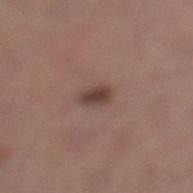  biopsy_status: not biopsied; imaged during a skin examination
  lesion_size:
    long_diameter_mm_approx: 2.5
  automated_metrics:
    cielab_L: 41
    cielab_a: 18
    cielab_b: 21
    vs_skin_darker_L: 11.0
  image:
    source: total-body photography crop
    field_of_view_mm: 15
  patient:
    sex: male
    age_approx: 45
  site: left lower leg
  lighting: white-light